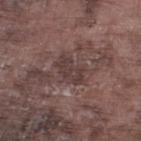The lesion's longest dimension is about 3.5 mm.
Automated tile analysis of the lesion measured an area of roughly 6.5 mm², an eccentricity of roughly 0.85, and a shape-asymmetry score of about 0.25 (0 = symmetric). It also reported a mean CIELAB color near L≈38 a*≈16 b*≈17 and a lesion-to-skin contrast of about 6.5 (normalized; higher = more distinct). The software also gave a classifier nevus-likeness of about 0/100 and a lesion-detection confidence of about 70/100.
The lesion is on the leg.
A roughly 15 mm field-of-view crop from a total-body skin photograph.
A male patient aged around 75.